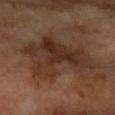follow-up — imaged on a skin check; not biopsied | lighting — cross-polarized illumination | location — the right forearm | subject — female, in their 60s | lesion diameter — ~9 mm (longest diameter) | image — ~15 mm tile from a whole-body skin photo.